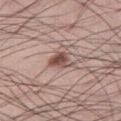workup — catalogued during a skin exam; not biopsied | anatomic site — the right thigh | image source — 15 mm crop, total-body photography | subject — male, aged around 55.